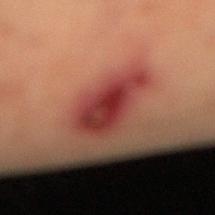notes = total-body-photography surveillance lesion; no biopsy.